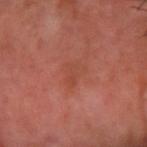Captured during whole-body skin photography for melanoma surveillance; the lesion was not biopsied. A male subject, aged around 60. A close-up tile cropped from a whole-body skin photograph, about 15 mm across. The lesion is located on the right forearm.